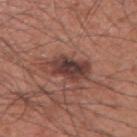| feature | finding |
|---|---|
| biopsy status | no biopsy performed (imaged during a skin exam) |
| patient | male, in their 60s |
| size | ≈5 mm |
| site | the right upper arm |
| image source | 15 mm crop, total-body photography |
| illumination | white-light |